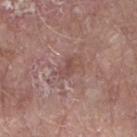Captured during whole-body skin photography for melanoma surveillance; the lesion was not biopsied.
Captured under white-light illumination.
From the arm.
The recorded lesion diameter is about 2.5 mm.
Automated image analysis of the tile measured a mean CIELAB color near L≈48 a*≈21 b*≈22 and roughly 7 lightness units darker than nearby skin. And it measured a classifier nevus-likeness of about 0/100 and lesion-presence confidence of about 90/100.
A 15 mm close-up tile from a total-body photography series done for melanoma screening.
The subject is a male aged 58 to 62.